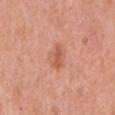<case>
  <automated_metrics>
    <shape_asymmetry>0.25</shape_asymmetry>
    <cielab_L>58</cielab_L>
    <cielab_a>28</cielab_a>
    <cielab_b>33</cielab_b>
    <vs_skin_darker_L>9.0</vs_skin_darker_L>
    <color_variation_0_10>1.5</color_variation_0_10>
    <peripheral_color_asymmetry>0.5</peripheral_color_asymmetry>
    <nevus_likeness_0_100>60</nevus_likeness_0_100>
    <lesion_detection_confidence_0_100>100</lesion_detection_confidence_0_100>
  </automated_metrics>
  <site>mid back</site>
  <image>
    <source>total-body photography crop</source>
    <field_of_view_mm>15</field_of_view_mm>
  </image>
  <patient>
    <sex>male</sex>
    <age_approx>70</age_approx>
  </patient>
  <lesion_size>
    <long_diameter_mm_approx>3.5</long_diameter_mm_approx>
  </lesion_size>
</case>